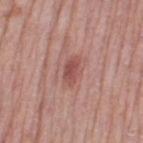Q: Was a biopsy performed?
A: imaged on a skin check; not biopsied
Q: What are the patient's age and sex?
A: female, about 65 years old
Q: How was this image acquired?
A: 15 mm crop, total-body photography
Q: What is the anatomic site?
A: the left thigh
Q: How large is the lesion?
A: ≈3 mm
Q: Automated lesion metrics?
A: a footprint of about 4 mm², an outline eccentricity of about 0.85 (0 = round, 1 = elongated), and a symmetry-axis asymmetry near 0.3; a lesion–skin lightness drop of about 10 and a normalized border contrast of about 7